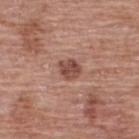<tbp_lesion>
<biopsy_status>not biopsied; imaged during a skin examination</biopsy_status>
<image>
  <source>total-body photography crop</source>
  <field_of_view_mm>15</field_of_view_mm>
</image>
<lighting>white-light</lighting>
<lesion_size>
  <long_diameter_mm_approx>2.5</long_diameter_mm_approx>
</lesion_size>
<site>upper back</site>
<patient>
  <sex>female</sex>
  <age_approx>60</age_approx>
</patient>
</tbp_lesion>